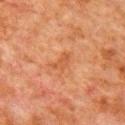| key | value |
|---|---|
| workup | imaged on a skin check; not biopsied |
| image | 15 mm crop, total-body photography |
| lighting | cross-polarized |
| subject | male, aged around 80 |
| size | ≈2.5 mm |
| location | the arm |